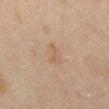Clinical impression: This lesion was catalogued during total-body skin photography and was not selected for biopsy. Acquisition and patient details: The subject is a male roughly 45 years of age. The lesion-visualizer software estimated a border-irregularity index near 3.5/10. Cropped from a whole-body photographic skin survey; the tile spans about 15 mm. On the abdomen. Captured under cross-polarized illumination.